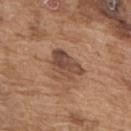Q: Was this lesion biopsied?
A: total-body-photography surveillance lesion; no biopsy
Q: What is the imaging modality?
A: ~15 mm tile from a whole-body skin photo
Q: What lighting was used for the tile?
A: white-light illumination
Q: Who is the patient?
A: male, roughly 75 years of age
Q: How large is the lesion?
A: about 4.5 mm
Q: Lesion location?
A: the upper back